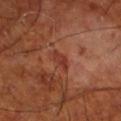The lesion was tiled from a total-body skin photograph and was not biopsied. The lesion is located on the left lower leg. A 15 mm crop from a total-body photograph taken for skin-cancer surveillance. A male subject, aged 68 to 72.